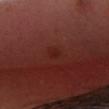Case summary:
– workup: catalogued during a skin exam; not biopsied
– image: ~15 mm tile from a whole-body skin photo
– size: about 2 mm
– location: the head or neck
– tile lighting: white-light illumination
– automated lesion analysis: a lesion area of about 2 mm² and an outline eccentricity of about 0.75 (0 = round, 1 = elongated); a lesion–skin lightness drop of about 4 and a normalized border contrast of about 4.5; a lesion-detection confidence of about 100/100
– patient: male, approximately 40 years of age